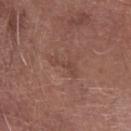No biopsy was performed on this lesion — it was imaged during a full skin examination and was not determined to be concerning. The lesion is on the right forearm. The lesion's longest dimension is about 3.5 mm. A roughly 15 mm field-of-view crop from a total-body skin photograph. A male subject roughly 65 years of age. The tile uses white-light illumination.